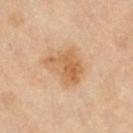The lesion was tiled from a total-body skin photograph and was not biopsied.
The tile uses cross-polarized illumination.
Approximately 4.5 mm at its widest.
The patient is female.
A 15 mm close-up tile from a total-body photography series done for melanoma screening.
The total-body-photography lesion software estimated an area of roughly 12 mm². It also reported border irregularity of about 3.5 on a 0–10 scale, a within-lesion color-variation index near 5.5/10, and peripheral color asymmetry of about 2.